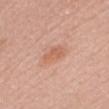<tbp_lesion>
  <biopsy_status>not biopsied; imaged during a skin examination</biopsy_status>
  <patient>
    <sex>female</sex>
    <age_approx>50</age_approx>
  </patient>
  <site>chest</site>
  <automated_metrics>
    <cielab_L>61</cielab_L>
    <cielab_a>25</cielab_a>
    <cielab_b>32</cielab_b>
    <vs_skin_darker_L>8.0</vs_skin_darker_L>
    <vs_skin_contrast_norm>5.5</vs_skin_contrast_norm>
  </automated_metrics>
  <lighting>white-light</lighting>
  <lesion_size>
    <long_diameter_mm_approx>3.5</long_diameter_mm_approx>
  </lesion_size>
  <image>
    <source>total-body photography crop</source>
    <field_of_view_mm>15</field_of_view_mm>
  </image>
</tbp_lesion>